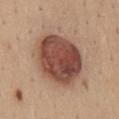The tile uses white-light illumination.
From the mid back.
A close-up tile cropped from a whole-body skin photograph, about 15 mm across.
A male subject about 35 years old.